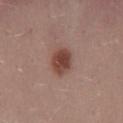  site: lower back
  image:
    source: total-body photography crop
    field_of_view_mm: 15
  lighting: white-light
  patient:
    sex: female
    age_approx: 25
  automated_metrics:
    area_mm2_approx: 7.0
    eccentricity: 0.7
    shape_asymmetry: 0.2
    cielab_L: 44
    cielab_a: 22
    cielab_b: 24
    vs_skin_darker_L: 13.0
    vs_skin_contrast_norm: 9.5
    border_irregularity_0_10: 2.0
    color_variation_0_10: 3.0
    peripheral_color_asymmetry: 1.0
  lesion_size:
    long_diameter_mm_approx: 3.5